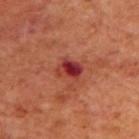Longest diameter approximately 3 mm. A male patient approximately 70 years of age. Cropped from a whole-body photographic skin survey; the tile spans about 15 mm. The lesion is located on the upper back. Captured under cross-polarized illumination.